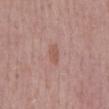Recorded during total-body skin imaging; not selected for excision or biopsy. The lesion is on the mid back. A 15 mm close-up extracted from a 3D total-body photography capture. This is a white-light tile. A male subject roughly 60 years of age. The lesion's longest dimension is about 3 mm.